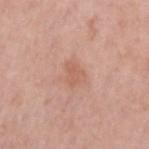<record>
<biopsy_status>not biopsied; imaged during a skin examination</biopsy_status>
<lesion_size>
  <long_diameter_mm_approx>3.0</long_diameter_mm_approx>
</lesion_size>
<site>left upper arm</site>
<image>
  <source>total-body photography crop</source>
  <field_of_view_mm>15</field_of_view_mm>
</image>
<patient>
  <sex>female</sex>
  <age_approx>40</age_approx>
</patient>
<lighting>white-light</lighting>
<automated_metrics>
  <area_mm2_approx>4.0</area_mm2_approx>
  <eccentricity>0.7</eccentricity>
  <shape_asymmetry>0.45</shape_asymmetry>
  <color_variation_0_10>1.0</color_variation_0_10>
  <peripheral_color_asymmetry>0.5</peripheral_color_asymmetry>
</automated_metrics>
</record>